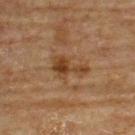Case summary:
– notes · no biopsy performed (imaged during a skin exam)
– lighting · cross-polarized
– image source · ~15 mm tile from a whole-body skin photo
– patient · male, aged around 65
– site · the back